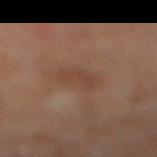Findings:
– notes: imaged on a skin check; not biopsied
– imaging modality: ~15 mm tile from a whole-body skin photo
– subject: male, aged around 65
– lesion size: about 3.5 mm
– automated metrics: a footprint of about 6.5 mm², an outline eccentricity of about 0.75 (0 = round, 1 = elongated), and a symmetry-axis asymmetry near 0.2; a lesion color around L≈41 a*≈17 b*≈27 in CIELAB, a lesion–skin lightness drop of about 5, and a normalized border contrast of about 5; a border-irregularity rating of about 2/10, a within-lesion color-variation index near 1.5/10, and peripheral color asymmetry of about 0.5; a nevus-likeness score of about 0/100 and lesion-presence confidence of about 100/100
– location: the leg
– lighting: cross-polarized illumination